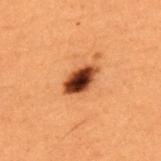Captured during whole-body skin photography for melanoma surveillance; the lesion was not biopsied. The subject is a male aged 48–52. The lesion-visualizer software estimated an area of roughly 6.5 mm², an eccentricity of roughly 0.8, and two-axis asymmetry of about 0.15. It also reported a classifier nevus-likeness of about 100/100 and a detector confidence of about 100 out of 100 that the crop contains a lesion. On the upper back. A roughly 15 mm field-of-view crop from a total-body skin photograph. Measured at roughly 4 mm in maximum diameter. Imaged with cross-polarized lighting.